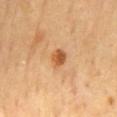Impression:
The lesion was photographed on a routine skin check and not biopsied; there is no pathology result.
Acquisition and patient details:
Cropped from a total-body skin-imaging series; the visible field is about 15 mm. The lesion's longest dimension is about 2 mm. The tile uses cross-polarized illumination. The lesion is located on the mid back. The patient is a male roughly 70 years of age.